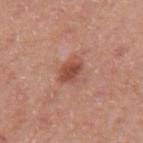Findings:
– biopsy status — no biopsy performed (imaged during a skin exam)
– site — the arm
– imaging modality — ~15 mm crop, total-body skin-cancer survey
– subject — female, aged approximately 40
– size — about 3.5 mm